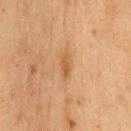A male subject in their mid- to late 70s.
From the front of the torso.
Automated tile analysis of the lesion measured an average lesion color of about L≈45 a*≈19 b*≈33 (CIELAB), a lesion–skin lightness drop of about 7, and a normalized border contrast of about 6. The software also gave a classifier nevus-likeness of about 40/100.
Cropped from a whole-body photographic skin survey; the tile spans about 15 mm.
Longest diameter approximately 3 mm.